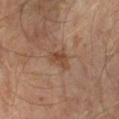follow-up: total-body-photography surveillance lesion; no biopsy | image-analysis metrics: an area of roughly 4.5 mm² and an outline eccentricity of about 0.65 (0 = round, 1 = elongated); a mean CIELAB color near L≈43 a*≈19 b*≈29, about 8 CIELAB-L* units darker than the surrounding skin, and a normalized lesion–skin contrast near 7; a detector confidence of about 100 out of 100 that the crop contains a lesion | acquisition: ~15 mm crop, total-body skin-cancer survey | subject: male, aged approximately 65 | illumination: cross-polarized | site: the left forearm | lesion diameter: ~3 mm (longest diameter).